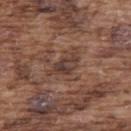Impression:
This lesion was catalogued during total-body skin photography and was not selected for biopsy.
Clinical summary:
Located on the upper back. A lesion tile, about 15 mm wide, cut from a 3D total-body photograph. Automated image analysis of the tile measured a lesion area of about 6.5 mm², an outline eccentricity of about 0.85 (0 = round, 1 = elongated), and a symmetry-axis asymmetry near 0.35. The software also gave border irregularity of about 4 on a 0–10 scale, internal color variation of about 6 on a 0–10 scale, and a peripheral color-asymmetry measure near 2. The patient is a male aged 73 to 77. Measured at roughly 4 mm in maximum diameter.